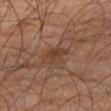Part of a total-body skin-imaging series; this lesion was reviewed on a skin check and was not flagged for biopsy. The lesion is located on the right thigh. A 15 mm close-up extracted from a 3D total-body photography capture. The total-body-photography lesion software estimated a footprint of about 6 mm², an eccentricity of roughly 0.6, and a shape-asymmetry score of about 0.25 (0 = symmetric). The software also gave roughly 6 lightness units darker than nearby skin and a normalized lesion–skin contrast near 6. The software also gave a border-irregularity rating of about 3/10, a color-variation rating of about 3/10, and peripheral color asymmetry of about 1. Captured under cross-polarized illumination. A male subject, aged approximately 55.